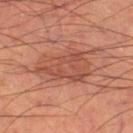{
  "biopsy_status": "not biopsied; imaged during a skin examination",
  "site": "left thigh",
  "automated_metrics": {
    "cielab_L": 44,
    "cielab_a": 24,
    "cielab_b": 28,
    "vs_skin_contrast_norm": 6.0
  },
  "patient": {
    "sex": "male",
    "age_approx": 55
  },
  "lesion_size": {
    "long_diameter_mm_approx": 6.0
  },
  "lighting": "cross-polarized",
  "image": {
    "source": "total-body photography crop",
    "field_of_view_mm": 15
  }
}